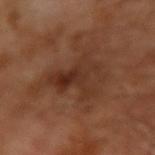This lesion was catalogued during total-body skin photography and was not selected for biopsy.
The recorded lesion diameter is about 5 mm.
A male subject roughly 65 years of age.
A 15 mm close-up extracted from a 3D total-body photography capture.
Imaged with cross-polarized lighting.